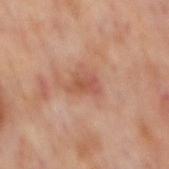| feature | finding |
|---|---|
| follow-up | no biopsy performed (imaged during a skin exam) |